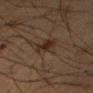Impression: No biopsy was performed on this lesion — it was imaged during a full skin examination and was not determined to be concerning. Background: This is a cross-polarized tile. A close-up tile cropped from a whole-body skin photograph, about 15 mm across. The recorded lesion diameter is about 3.5 mm. A male patient, aged 53 to 57. On the right forearm.